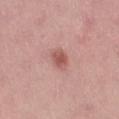workup: catalogued during a skin exam; not biopsied
body site: the left thigh
tile lighting: white-light
diameter: ≈2.5 mm
image source: ~15 mm tile from a whole-body skin photo
patient: female, aged approximately 40